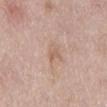workup = no biopsy performed (imaged during a skin exam) | illumination = white-light illumination | subject = male, aged 53 to 57 | diameter = ~3 mm (longest diameter) | image-analysis metrics = a mean CIELAB color near L≈61 a*≈18 b*≈29 and a lesion–skin lightness drop of about 7; a border-irregularity rating of about 3.5/10, internal color variation of about 2 on a 0–10 scale, and radial color variation of about 0.5 | acquisition = total-body-photography crop, ~15 mm field of view | body site = the mid back.